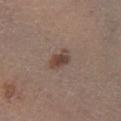| feature | finding |
|---|---|
| follow-up | no biopsy performed (imaged during a skin exam) |
| anatomic site | the left lower leg |
| automated metrics | an average lesion color of about L≈43 a*≈16 b*≈22 (CIELAB), a lesion–skin lightness drop of about 10, and a normalized border contrast of about 8; a color-variation rating of about 4/10 and radial color variation of about 1 |
| lighting | white-light |
| imaging modality | total-body-photography crop, ~15 mm field of view |
| subject | male, aged around 55 |
| lesion size | ~3 mm (longest diameter) |